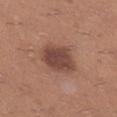workup = total-body-photography surveillance lesion; no biopsy
image = 15 mm crop, total-body photography
body site = the right lower leg
subject = male, roughly 40 years of age